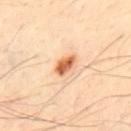Impression:
The lesion was photographed on a routine skin check and not biopsied; there is no pathology result.
Image and clinical context:
A male patient, roughly 50 years of age. Captured under cross-polarized illumination. A 15 mm close-up tile from a total-body photography series done for melanoma screening. From the upper back. Approximately 3 mm at its widest.